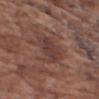Clinical impression:
Captured during whole-body skin photography for melanoma surveillance; the lesion was not biopsied.
Image and clinical context:
Captured under white-light illumination. About 3.5 mm across. A male patient, in their mid- to late 70s. A 15 mm close-up extracted from a 3D total-body photography capture. From the right upper arm. The total-body-photography lesion software estimated two-axis asymmetry of about 0.2. The analysis additionally found a border-irregularity index near 2.5/10 and radial color variation of about 1.